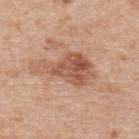<lesion>
  <site>upper back</site>
  <patient>
    <sex>male</sex>
    <age_approx>70</age_approx>
  </patient>
  <image>
    <source>total-body photography crop</source>
    <field_of_view_mm>15</field_of_view_mm>
  </image>
</lesion>